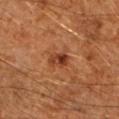• illumination — cross-polarized
• site — the right upper arm
• size — ≈2.5 mm
• image source — total-body-photography crop, ~15 mm field of view
• subject — male, in their 60s